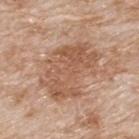Recorded during total-body skin imaging; not selected for excision or biopsy.
A roughly 15 mm field-of-view crop from a total-body skin photograph.
The recorded lesion diameter is about 7.5 mm.
From the upper back.
The tile uses white-light illumination.
A male patient in their 80s.